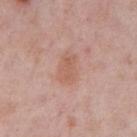workup: no biopsy performed (imaged during a skin exam) | automated lesion analysis: an area of roughly 8.5 mm² and a symmetry-axis asymmetry near 0.2; a border-irregularity rating of about 2/10, a color-variation rating of about 2.5/10, and radial color variation of about 1 | image: total-body-photography crop, ~15 mm field of view | subject: male, approximately 75 years of age | tile lighting: white-light | anatomic site: the chest | lesion size: ~4 mm (longest diameter).